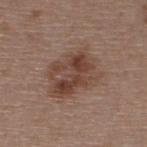  biopsy_status: not biopsied; imaged during a skin examination
  automated_metrics:
    eccentricity: 0.65
    shape_asymmetry: 0.25
    border_irregularity_0_10: 3.0
    color_variation_0_10: 7.0
    peripheral_color_asymmetry: 2.5
  patient:
    sex: female
    age_approx: 45
  image:
    source: total-body photography crop
    field_of_view_mm: 15
  lesion_size:
    long_diameter_mm_approx: 6.5
  lighting: white-light
  site: upper back